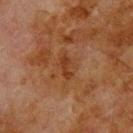Assessment:
Part of a total-body skin-imaging series; this lesion was reviewed on a skin check and was not flagged for biopsy.
Clinical summary:
This is a cross-polarized tile. Cropped from a whole-body photographic skin survey; the tile spans about 15 mm. The lesion is on the upper back. Measured at roughly 3 mm in maximum diameter. The total-body-photography lesion software estimated a lesion color around L≈29 a*≈20 b*≈29 in CIELAB and a lesion–skin lightness drop of about 6. The analysis additionally found a border-irregularity rating of about 3.5/10, a within-lesion color-variation index near 0/10, and peripheral color asymmetry of about 0. The software also gave a nevus-likeness score of about 0/100 and lesion-presence confidence of about 100/100. A male patient, aged 78 to 82.